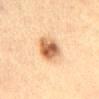Q: Was a biopsy performed?
A: catalogued during a skin exam; not biopsied
Q: Automated lesion metrics?
A: an area of roughly 10 mm², an outline eccentricity of about 0.85 (0 = round, 1 = elongated), and a shape-asymmetry score of about 0.15 (0 = symmetric); a lesion–skin lightness drop of about 15; a border-irregularity rating of about 2/10, a within-lesion color-variation index near 7/10, and radial color variation of about 2
Q: Lesion size?
A: ≈5 mm
Q: Lesion location?
A: the mid back
Q: Who is the patient?
A: female, aged 63–67
Q: What is the imaging modality?
A: 15 mm crop, total-body photography
Q: Illumination type?
A: cross-polarized illumination